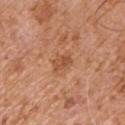No biopsy was performed on this lesion — it was imaged during a full skin examination and was not determined to be concerning.
The lesion-visualizer software estimated a lesion area of about 4 mm², a shape eccentricity near 0.75, and two-axis asymmetry of about 0.2. And it measured a mean CIELAB color near L≈52 a*≈25 b*≈36, about 9 CIELAB-L* units darker than the surrounding skin, and a normalized border contrast of about 6.5. The analysis additionally found a border-irregularity index near 2/10 and a within-lesion color-variation index near 3/10.
The recorded lesion diameter is about 2.5 mm.
The lesion is located on the left upper arm.
Imaged with white-light lighting.
The subject is a male aged around 75.
Cropped from a total-body skin-imaging series; the visible field is about 15 mm.